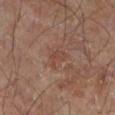No biopsy was performed on this lesion — it was imaged during a full skin examination and was not determined to be concerning. Measured at roughly 3 mm in maximum diameter. A male subject aged around 65. On the left lower leg. The total-body-photography lesion software estimated a lesion area of about 3 mm² and two-axis asymmetry of about 0.45. And it measured a mean CIELAB color near L≈42 a*≈19 b*≈26, roughly 5 lightness units darker than nearby skin, and a lesion-to-skin contrast of about 4.5 (normalized; higher = more distinct). Cropped from a total-body skin-imaging series; the visible field is about 15 mm. Captured under cross-polarized illumination.